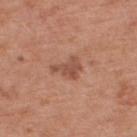Clinical impression:
The lesion was tiled from a total-body skin photograph and was not biopsied.
Clinical summary:
A lesion tile, about 15 mm wide, cut from a 3D total-body photograph. The patient is a male about 70 years old. The tile uses white-light illumination. An algorithmic analysis of the crop reported a lesion area of about 5.5 mm², an outline eccentricity of about 0.75 (0 = round, 1 = elongated), and two-axis asymmetry of about 0.55. It also reported a lesion color around L≈51 a*≈23 b*≈29 in CIELAB, a lesion–skin lightness drop of about 10, and a normalized lesion–skin contrast near 7. The analysis additionally found a border-irregularity index near 5.5/10 and radial color variation of about 0.5. The analysis additionally found an automated nevus-likeness rating near 0 out of 100 and a detector confidence of about 100 out of 100 that the crop contains a lesion. The lesion's longest dimension is about 3.5 mm. The lesion is located on the upper back.